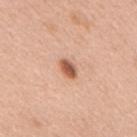| key | value |
|---|---|
| notes | no biopsy performed (imaged during a skin exam) |
| anatomic site | the upper back |
| image | ~15 mm tile from a whole-body skin photo |
| subject | female, aged 43 to 47 |
| automated metrics | a symmetry-axis asymmetry near 0.15; a mean CIELAB color near L≈58 a*≈24 b*≈33, about 15 CIELAB-L* units darker than the surrounding skin, and a normalized lesion–skin contrast near 9.5; a border-irregularity index near 1.5/10 and peripheral color asymmetry of about 1 |